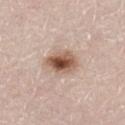The lesion was photographed on a routine skin check and not biopsied; there is no pathology result.
A male subject in their 80s.
The lesion is located on the left thigh.
Measured at roughly 4 mm in maximum diameter.
Cropped from a whole-body photographic skin survey; the tile spans about 15 mm.